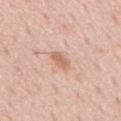This lesion was catalogued during total-body skin photography and was not selected for biopsy. About 3 mm across. Captured under white-light illumination. On the chest. A male patient aged 53–57. A lesion tile, about 15 mm wide, cut from a 3D total-body photograph.